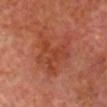follow-up = no biopsy performed (imaged during a skin exam); patient = male, in their mid-60s; acquisition = 15 mm crop, total-body photography; location = the head or neck; lesion diameter = about 6.5 mm; TBP lesion metrics = an automated nevus-likeness rating near 0 out of 100 and lesion-presence confidence of about 100/100.